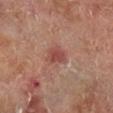The lesion was tiled from a total-body skin photograph and was not biopsied.